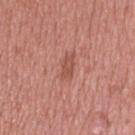Q: Is there a histopathology result?
A: total-body-photography surveillance lesion; no biopsy
Q: What is the anatomic site?
A: the upper back
Q: What is the imaging modality?
A: total-body-photography crop, ~15 mm field of view
Q: Automated lesion metrics?
A: a lesion color around L≈52 a*≈27 b*≈28 in CIELAB, a lesion–skin lightness drop of about 8, and a normalized lesion–skin contrast near 6; border irregularity of about 2.5 on a 0–10 scale and a peripheral color-asymmetry measure near 0.5
Q: Lesion size?
A: about 3 mm
Q: What lighting was used for the tile?
A: white-light
Q: Patient demographics?
A: male, in their mid-30s